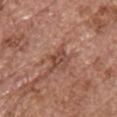This lesion was catalogued during total-body skin photography and was not selected for biopsy.
The recorded lesion diameter is about 2.5 mm.
This is a white-light tile.
A female patient in their mid- to late 60s.
A roughly 15 mm field-of-view crop from a total-body skin photograph.
The lesion is on the chest.
Automated image analysis of the tile measured a lesion area of about 3 mm², an outline eccentricity of about 0.8 (0 = round, 1 = elongated), and a shape-asymmetry score of about 0.35 (0 = symmetric). And it measured a lesion color around L≈45 a*≈23 b*≈28 in CIELAB, roughly 9 lightness units darker than nearby skin, and a lesion-to-skin contrast of about 7 (normalized; higher = more distinct). And it measured a border-irregularity rating of about 3.5/10 and peripheral color asymmetry of about 0.5. It also reported a detector confidence of about 65 out of 100 that the crop contains a lesion.